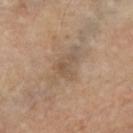The lesion was photographed on a routine skin check and not biopsied; there is no pathology result. Captured under cross-polarized illumination. A 15 mm close-up extracted from a 3D total-body photography capture. The lesion is located on the arm. The patient is a female aged approximately 65. The recorded lesion diameter is about 3 mm.